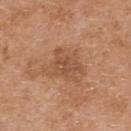This lesion was catalogued during total-body skin photography and was not selected for biopsy. About 4.5 mm across. The lesion is located on the upper back. The tile uses white-light illumination. Automated image analysis of the tile measured an outline eccentricity of about 0.6 (0 = round, 1 = elongated) and two-axis asymmetry of about 0.45. And it measured roughly 8 lightness units darker than nearby skin and a normalized lesion–skin contrast near 6. And it measured a within-lesion color-variation index near 3/10 and peripheral color asymmetry of about 1. The software also gave a nevus-likeness score of about 0/100. A female subject, aged 38–42. Cropped from a total-body skin-imaging series; the visible field is about 15 mm.